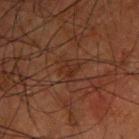Q: Is there a histopathology result?
A: imaged on a skin check; not biopsied
Q: Where on the body is the lesion?
A: the front of the torso
Q: What kind of image is this?
A: 15 mm crop, total-body photography
Q: How was the tile lit?
A: cross-polarized
Q: Who is the patient?
A: male, aged 48 to 52